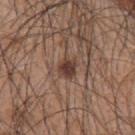  biopsy_status: not biopsied; imaged during a skin examination
  site: upper back
  image:
    source: total-body photography crop
    field_of_view_mm: 15
  patient:
    sex: male
    age_approx: 45
  automated_metrics:
    border_irregularity_0_10: 2.0
    color_variation_0_10: 2.5
    peripheral_color_asymmetry: 1.0
    nevus_likeness_0_100: 95
    lesion_detection_confidence_0_100: 100
  lighting: white-light
  lesion_size:
    long_diameter_mm_approx: 3.0